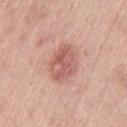notes=imaged on a skin check; not biopsied | patient=female, in their mid-50s | image-analysis metrics=an eccentricity of roughly 0.65; a lesion color around L≈59 a*≈25 b*≈25 in CIELAB and a normalized lesion–skin contrast near 7; a border-irregularity rating of about 1.5/10, a within-lesion color-variation index near 4.5/10, and radial color variation of about 1.5; a classifier nevus-likeness of about 25/100 | anatomic site=the left thigh | imaging modality=~15 mm crop, total-body skin-cancer survey | size=~4.5 mm (longest diameter).